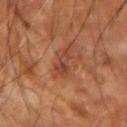  biopsy_status: not biopsied; imaged during a skin examination
  image:
    source: total-body photography crop
    field_of_view_mm: 15
  site: arm
  lighting: cross-polarized
  patient:
    age_approx: 65
  lesion_size:
    long_diameter_mm_approx: 3.5